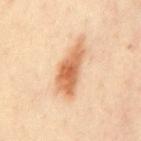Case summary:
• tile lighting: cross-polarized illumination
• TBP lesion metrics: a mean CIELAB color near L≈67 a*≈23 b*≈39, roughly 14 lightness units darker than nearby skin, and a lesion-to-skin contrast of about 9 (normalized; higher = more distinct); a border-irregularity rating of about 4/10 and radial color variation of about 1.5
• acquisition: ~15 mm tile from a whole-body skin photo
• anatomic site: the chest
• size: about 7 mm
• patient: male, approximately 50 years of age